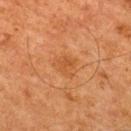A male patient approximately 65 years of age.
On the upper back.
Cropped from a whole-body photographic skin survey; the tile spans about 15 mm.